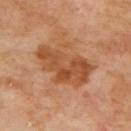Located on the back. A roughly 15 mm field-of-view crop from a total-body skin photograph. A male subject aged 68 to 72.